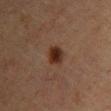Notes:
- follow-up — catalogued during a skin exam; not biopsied
- imaging modality — 15 mm crop, total-body photography
- lighting — cross-polarized
- patient — female, aged 63–67
- anatomic site — the chest
- automated metrics — border irregularity of about 1.5 on a 0–10 scale and a peripheral color-asymmetry measure near 1.5